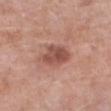Assessment:
No biopsy was performed on this lesion — it was imaged during a full skin examination and was not determined to be concerning.
Image and clinical context:
The lesion is located on the left lower leg. The subject is a male roughly 80 years of age. The recorded lesion diameter is about 4 mm. Automated tile analysis of the lesion measured a mean CIELAB color near L≈51 a*≈24 b*≈27, a lesion–skin lightness drop of about 11, and a lesion-to-skin contrast of about 8 (normalized; higher = more distinct). The analysis additionally found a border-irregularity index near 2.5/10 and peripheral color asymmetry of about 1. And it measured a nevus-likeness score of about 70/100 and a detector confidence of about 100 out of 100 that the crop contains a lesion. This is a white-light tile. A roughly 15 mm field-of-view crop from a total-body skin photograph.